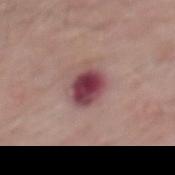| feature | finding |
|---|---|
| follow-up | total-body-photography surveillance lesion; no biopsy |
| acquisition | ~15 mm crop, total-body skin-cancer survey |
| subject | male, in their 70s |
| body site | the mid back |
| lesion size | ~3.5 mm (longest diameter) |